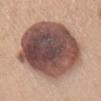Assessment:
This lesion was catalogued during total-body skin photography and was not selected for biopsy.
Background:
A female subject, aged 38–42. Measured at roughly 11 mm in maximum diameter. The lesion is located on the front of the torso. A region of skin cropped from a whole-body photographic capture, roughly 15 mm wide.